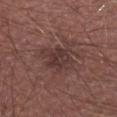Assessment: Recorded during total-body skin imaging; not selected for excision or biopsy. Clinical summary: Measured at roughly 4.5 mm in maximum diameter. On the arm. The subject is a male aged 58–62. Cropped from a total-body skin-imaging series; the visible field is about 15 mm. Imaged with white-light lighting.